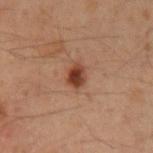Assessment: Imaged during a routine full-body skin examination; the lesion was not biopsied and no histopathology is available. Clinical summary: Approximately 2.5 mm at its widest. A region of skin cropped from a whole-body photographic capture, roughly 15 mm wide. A male patient in their 50s. The lesion is on the arm.